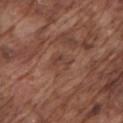<tbp_lesion>
<biopsy_status>not biopsied; imaged during a skin examination</biopsy_status>
<site>arm</site>
<image>
  <source>total-body photography crop</source>
  <field_of_view_mm>15</field_of_view_mm>
</image>
<lighting>white-light</lighting>
<lesion_size>
  <long_diameter_mm_approx>2.5</long_diameter_mm_approx>
</lesion_size>
<patient>
  <sex>male</sex>
  <age_approx>75</age_approx>
</patient>
</tbp_lesion>